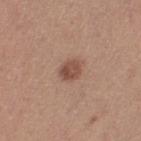Assessment:
The lesion was tiled from a total-body skin photograph and was not biopsied.
Background:
The lesion is on the right thigh. This is a white-light tile. Approximately 2.5 mm at its widest. A 15 mm crop from a total-body photograph taken for skin-cancer surveillance. The total-body-photography lesion software estimated a lesion area of about 4.5 mm², an eccentricity of roughly 0.6, and two-axis asymmetry of about 0.2. The software also gave a within-lesion color-variation index near 3.5/10. The analysis additionally found a nevus-likeness score of about 90/100 and a lesion-detection confidence of about 100/100. A female subject, aged 53–57.